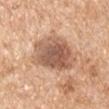Imaged during a routine full-body skin examination; the lesion was not biopsied and no histopathology is available.
About 6.5 mm across.
A 15 mm close-up extracted from a 3D total-body photography capture.
The total-body-photography lesion software estimated a lesion color around L≈58 a*≈20 b*≈31 in CIELAB, roughly 14 lightness units darker than nearby skin, and a normalized lesion–skin contrast near 9. And it measured a border-irregularity rating of about 2.5/10, a color-variation rating of about 6/10, and a peripheral color-asymmetry measure near 1.5. The software also gave a classifier nevus-likeness of about 5/100 and lesion-presence confidence of about 100/100.
From the arm.
A male subject, in their 60s.